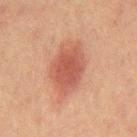Notes:
– image source — ~15 mm crop, total-body skin-cancer survey
– anatomic site — the chest
– subject — male, in their mid-60s
– lighting — cross-polarized illumination